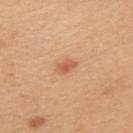workup: catalogued during a skin exam; not biopsied | site: the back | subject: female, approximately 30 years of age | image: ~15 mm crop, total-body skin-cancer survey | lesion size: ≈2.5 mm | automated lesion analysis: a lesion area of about 3.5 mm² and two-axis asymmetry of about 0.25; a classifier nevus-likeness of about 80/100 and lesion-presence confidence of about 100/100.